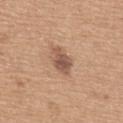Findings:
• follow-up: no biopsy performed (imaged during a skin exam)
• patient: male, in their mid- to late 60s
• lesion diameter: ~3.5 mm (longest diameter)
• imaging modality: total-body-photography crop, ~15 mm field of view
• image-analysis metrics: an average lesion color of about L≈53 a*≈19 b*≈29 (CIELAB), a lesion–skin lightness drop of about 12, and a normalized border contrast of about 8.5; border irregularity of about 3 on a 0–10 scale, a within-lesion color-variation index near 2.5/10, and a peripheral color-asymmetry measure near 1
• location: the back